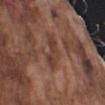This is a white-light tile. From the left upper arm. A male patient, aged 73 to 77. A 15 mm close-up extracted from a 3D total-body photography capture.